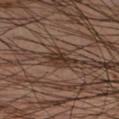{
  "biopsy_status": "not biopsied; imaged during a skin examination",
  "automated_metrics": {
    "area_mm2_approx": 6.0,
    "eccentricity": 0.8,
    "shape_asymmetry": 0.25,
    "vs_skin_contrast_norm": 9.0,
    "border_irregularity_0_10": 3.0,
    "color_variation_0_10": 4.0,
    "peripheral_color_asymmetry": 1.5
  },
  "image": {
    "source": "total-body photography crop",
    "field_of_view_mm": 15
  },
  "patient": {
    "sex": "male",
    "age_approx": 50
  },
  "lighting": "cross-polarized",
  "site": "leg",
  "lesion_size": {
    "long_diameter_mm_approx": 4.0
  }
}